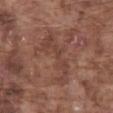Q: Was a biopsy performed?
A: catalogued during a skin exam; not biopsied
Q: Lesion location?
A: the abdomen
Q: Patient demographics?
A: male, approximately 75 years of age
Q: What did automated image analysis measure?
A: a mean CIELAB color near L≈42 a*≈21 b*≈25, roughly 6 lightness units darker than nearby skin, and a lesion-to-skin contrast of about 5 (normalized; higher = more distinct)
Q: How large is the lesion?
A: ≈6.5 mm
Q: What kind of image is this?
A: ~15 mm crop, total-body skin-cancer survey
Q: What lighting was used for the tile?
A: white-light illumination